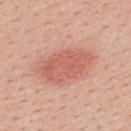Q: Automated lesion metrics?
A: a mean CIELAB color near L≈60 a*≈29 b*≈30, about 10 CIELAB-L* units darker than the surrounding skin, and a normalized lesion–skin contrast near 6.5; a border-irregularity index near 2/10, internal color variation of about 2.5 on a 0–10 scale, and a peripheral color-asymmetry measure near 1; lesion-presence confidence of about 100/100
Q: How was this image acquired?
A: ~15 mm tile from a whole-body skin photo
Q: How was the tile lit?
A: white-light illumination
Q: What are the patient's age and sex?
A: female, aged around 40
Q: Lesion size?
A: ~6.5 mm (longest diameter)
Q: Lesion location?
A: the upper back